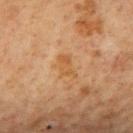Captured during whole-body skin photography for melanoma surveillance; the lesion was not biopsied. The subject is a male about 65 years old. A close-up tile cropped from a whole-body skin photograph, about 15 mm across. The lesion is located on the mid back.